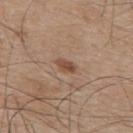{"biopsy_status": "not biopsied; imaged during a skin examination", "image": {"source": "total-body photography crop", "field_of_view_mm": 15}, "automated_metrics": {"cielab_L": 48, "cielab_a": 19, "cielab_b": 29, "vs_skin_contrast_norm": 7.5, "border_irregularity_0_10": 2.5, "color_variation_0_10": 1.5, "peripheral_color_asymmetry": 0.5, "nevus_likeness_0_100": 85, "lesion_detection_confidence_0_100": 100}, "lesion_size": {"long_diameter_mm_approx": 2.5}, "site": "mid back", "patient": {"sex": "male", "age_approx": 75}}